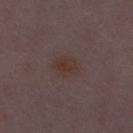Clinical impression:
Recorded during total-body skin imaging; not selected for excision or biopsy.
Context:
A female patient aged around 30. The tile uses white-light illumination. The recorded lesion diameter is about 3 mm. A roughly 15 mm field-of-view crop from a total-body skin photograph. From the leg. An algorithmic analysis of the crop reported an area of roughly 6 mm², an outline eccentricity of about 0.65 (0 = round, 1 = elongated), and two-axis asymmetry of about 0.15. It also reported a mean CIELAB color near L≈33 a*≈15 b*≈18 and a normalized lesion–skin contrast near 6. And it measured an automated nevus-likeness rating near 45 out of 100 and a lesion-detection confidence of about 100/100.